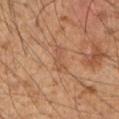Notes:
• workup · catalogued during a skin exam; not biopsied
• automated metrics · a footprint of about 3 mm², an outline eccentricity of about 0.95 (0 = round, 1 = elongated), and a symmetry-axis asymmetry near 0.4; roughly 6 lightness units darker than nearby skin and a normalized border contrast of about 5
• diameter · ≈3.5 mm
• image source · ~15 mm crop, total-body skin-cancer survey
• anatomic site · the right upper arm
• subject · male, aged 48–52